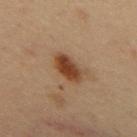workup: catalogued during a skin exam; not biopsied
subject: male, aged 53 to 57
body site: the mid back
image: ~15 mm crop, total-body skin-cancer survey
size: ≈4 mm